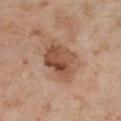<lesion>
  <biopsy_status>not biopsied; imaged during a skin examination</biopsy_status>
  <image>
    <source>total-body photography crop</source>
    <field_of_view_mm>15</field_of_view_mm>
  </image>
  <site>left thigh</site>
  <patient>
    <sex>female</sex>
    <age_approx>55</age_approx>
  </patient>
</lesion>